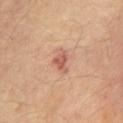Part of a total-body skin-imaging series; this lesion was reviewed on a skin check and was not flagged for biopsy.
The total-body-photography lesion software estimated a lesion area of about 4.5 mm², an outline eccentricity of about 0.75 (0 = round, 1 = elongated), and two-axis asymmetry of about 0.35. And it measured a mean CIELAB color near L≈56 a*≈24 b*≈28, a lesion–skin lightness drop of about 10, and a lesion-to-skin contrast of about 7 (normalized; higher = more distinct).
On the chest.
Captured under cross-polarized illumination.
The patient is a male aged 83 or over.
This image is a 15 mm lesion crop taken from a total-body photograph.